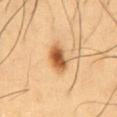The lesion was photographed on a routine skin check and not biopsied; there is no pathology result. A male subject in their 60s. Automated tile analysis of the lesion measured an area of roughly 7 mm², an outline eccentricity of about 0.8 (0 = round, 1 = elongated), and a symmetry-axis asymmetry near 0.25. The tile uses cross-polarized illumination. A 15 mm close-up extracted from a 3D total-body photography capture. From the abdomen.